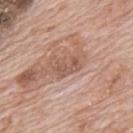<lesion>
<biopsy_status>not biopsied; imaged during a skin examination</biopsy_status>
<lesion_size>
  <long_diameter_mm_approx>3.5</long_diameter_mm_approx>
</lesion_size>
<site>upper back</site>
<image>
  <source>total-body photography crop</source>
  <field_of_view_mm>15</field_of_view_mm>
</image>
<patient>
  <sex>male</sex>
  <age_approx>70</age_approx>
</patient>
</lesion>